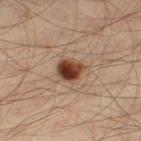follow-up = imaged on a skin check; not biopsied
TBP lesion metrics = an area of roughly 6.5 mm², an eccentricity of roughly 0.55, and a symmetry-axis asymmetry near 0.2; a mean CIELAB color near L≈37 a*≈20 b*≈28 and roughly 17 lightness units darker than nearby skin
diameter = about 3 mm
location = the left thigh
acquisition = 15 mm crop, total-body photography
patient = male, aged 33 to 37